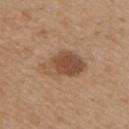Q: Was a biopsy performed?
A: no biopsy performed (imaged during a skin exam)
Q: How large is the lesion?
A: ~5.5 mm (longest diameter)
Q: Lesion location?
A: the front of the torso
Q: What are the patient's age and sex?
A: male, in their 50s
Q: What is the imaging modality?
A: 15 mm crop, total-body photography
Q: Illumination type?
A: white-light illumination
Q: What did automated image analysis measure?
A: a shape-asymmetry score of about 0.25 (0 = symmetric)